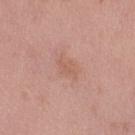The lesion was tiled from a total-body skin photograph and was not biopsied.